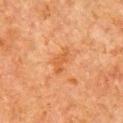Impression:
Part of a total-body skin-imaging series; this lesion was reviewed on a skin check and was not flagged for biopsy.
Background:
A 15 mm close-up extracted from a 3D total-body photography capture. On the right upper arm. The subject is a male aged 78–82. Approximately 3.5 mm at its widest.